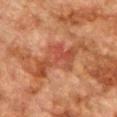Clinical impression: The lesion was photographed on a routine skin check and not biopsied; there is no pathology result. Image and clinical context: Approximately 8 mm at its widest. On the front of the torso. Imaged with cross-polarized lighting. Cropped from a whole-body photographic skin survey; the tile spans about 15 mm. A male patient aged around 75.